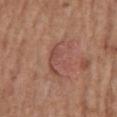biopsy status: catalogued during a skin exam; not biopsied
patient: male, in their mid-70s
lighting: white-light illumination
acquisition: 15 mm crop, total-body photography
body site: the mid back
diameter: ≈4.5 mm